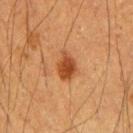<lesion>
  <image>
    <source>total-body photography crop</source>
    <field_of_view_mm>15</field_of_view_mm>
  </image>
  <site>right upper arm</site>
  <automated_metrics>
    <area_mm2_approx>6.5</area_mm2_approx>
    <shape_asymmetry>0.3</shape_asymmetry>
    <vs_skin_darker_L>12.0</vs_skin_darker_L>
    <vs_skin_contrast_norm>9.0</vs_skin_contrast_norm>
    <border_irregularity_0_10>2.5</border_irregularity_0_10>
    <color_variation_0_10>3.5</color_variation_0_10>
    <peripheral_color_asymmetry>1.0</peripheral_color_asymmetry>
  </automated_metrics>
  <patient>
    <sex>male</sex>
    <age_approx>60</age_approx>
  </patient>
  <lesion_size>
    <long_diameter_mm_approx>3.5</long_diameter_mm_approx>
  </lesion_size>
</lesion>